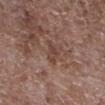{
  "biopsy_status": "not biopsied; imaged during a skin examination",
  "site": "right lower leg",
  "image": {
    "source": "total-body photography crop",
    "field_of_view_mm": 15
  },
  "lighting": "white-light",
  "patient": {
    "sex": "male",
    "age_approx": 70
  },
  "automated_metrics": {
    "area_mm2_approx": 4.0,
    "eccentricity": 0.75,
    "shape_asymmetry": 0.45,
    "nevus_likeness_0_100": 0,
    "lesion_detection_confidence_0_100": 90
  },
  "lesion_size": {
    "long_diameter_mm_approx": 3.0
  }
}